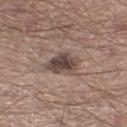| key | value |
|---|---|
| follow-up | imaged on a skin check; not biopsied |
| tile lighting | white-light |
| diameter | ≈4 mm |
| image source | ~15 mm crop, total-body skin-cancer survey |
| anatomic site | the right thigh |
| subject | male, about 40 years old |